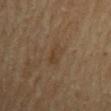Part of a total-body skin-imaging series; this lesion was reviewed on a skin check and was not flagged for biopsy. The lesion is on the mid back. Measured at roughly 3.5 mm in maximum diameter. The patient is a male approximately 70 years of age. A 15 mm close-up extracted from a 3D total-body photography capture. An algorithmic analysis of the crop reported a border-irregularity index near 4/10, a color-variation rating of about 2.5/10, and peripheral color asymmetry of about 1. Imaged with cross-polarized lighting.